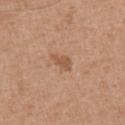follow-up: imaged on a skin check; not biopsied | subject: male, aged 53–57 | imaging modality: 15 mm crop, total-body photography | lesion diameter: about 3 mm | automated lesion analysis: a footprint of about 3.5 mm² and a symmetry-axis asymmetry near 0.3 | illumination: white-light illumination | body site: the chest.